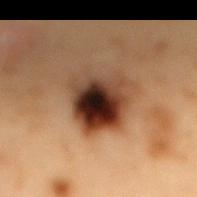Clinical impression:
Recorded during total-body skin imaging; not selected for excision or biopsy.
Clinical summary:
A 15 mm close-up tile from a total-body photography series done for melanoma screening. A male subject, approximately 55 years of age. From the mid back. This is a cross-polarized tile. The recorded lesion diameter is about 5.5 mm.